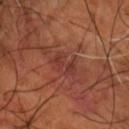{
  "lighting": "cross-polarized",
  "automated_metrics": {
    "eccentricity": 0.8
  },
  "image": {
    "source": "total-body photography crop",
    "field_of_view_mm": 15
  },
  "site": "right forearm",
  "patient": {
    "sex": "male",
    "age_approx": 55
  }
}